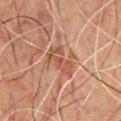Clinical impression: The lesion was tiled from a total-body skin photograph and was not biopsied. Clinical summary: Automated image analysis of the tile measured an average lesion color of about L≈41 a*≈20 b*≈26 (CIELAB), a lesion–skin lightness drop of about 7, and a normalized lesion–skin contrast near 6.5. It also reported a nevus-likeness score of about 0/100. The recorded lesion diameter is about 4 mm. The lesion is located on the chest. The subject is a male in their 50s. A 15 mm close-up tile from a total-body photography series done for melanoma screening. Captured under cross-polarized illumination.